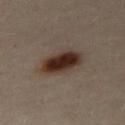<record>
<biopsy_status>not biopsied; imaged during a skin examination</biopsy_status>
<image>
  <source>total-body photography crop</source>
  <field_of_view_mm>15</field_of_view_mm>
</image>
<site>leg</site>
<patient>
  <sex>female</sex>
  <age_approx>40</age_approx>
</patient>
</record>